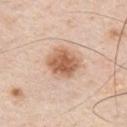Imaged during a routine full-body skin examination; the lesion was not biopsied and no histopathology is available. From the chest. Automated image analysis of the tile measured a footprint of about 13 mm², an eccentricity of roughly 0.5, and a shape-asymmetry score of about 0.15 (0 = symmetric). It also reported a lesion color around L≈62 a*≈21 b*≈32 in CIELAB, a lesion–skin lightness drop of about 14, and a normalized border contrast of about 9. The analysis additionally found a border-irregularity index near 2/10, internal color variation of about 5 on a 0–10 scale, and peripheral color asymmetry of about 1.5. Imaged with white-light lighting. Approximately 4.5 mm at its widest. This image is a 15 mm lesion crop taken from a total-body photograph. The subject is a male aged approximately 45.